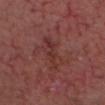biopsy status — imaged on a skin check; not biopsied